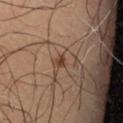A male subject, aged 63–67. A roughly 15 mm field-of-view crop from a total-body skin photograph. From the right thigh.